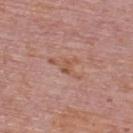biopsy status: catalogued during a skin exam; not biopsied
anatomic site: the upper back
lesion diameter: ≈4.5 mm
subject: male, roughly 75 years of age
image-analysis metrics: a lesion color around L≈55 a*≈22 b*≈29 in CIELAB, about 6 CIELAB-L* units darker than the surrounding skin, and a lesion-to-skin contrast of about 6 (normalized; higher = more distinct); a border-irregularity index near 6.5/10, a within-lesion color-variation index near 3/10, and a peripheral color-asymmetry measure near 1
imaging modality: 15 mm crop, total-body photography